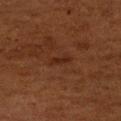{
  "biopsy_status": "not biopsied; imaged during a skin examination",
  "lighting": "cross-polarized",
  "image": {
    "source": "total-body photography crop",
    "field_of_view_mm": 15
  },
  "patient": {
    "sex": "male",
    "age_approx": 60
  },
  "site": "left upper arm",
  "automated_metrics": {
    "nevus_likeness_0_100": 5,
    "lesion_detection_confidence_0_100": 100
  }
}